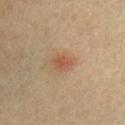<tbp_lesion>
<biopsy_status>not biopsied; imaged during a skin examination</biopsy_status>
<site>arm</site>
<lesion_size>
  <long_diameter_mm_approx>2.5</long_diameter_mm_approx>
</lesion_size>
<image>
  <source>total-body photography crop</source>
  <field_of_view_mm>15</field_of_view_mm>
</image>
<lighting>cross-polarized</lighting>
<patient>
  <sex>male</sex>
  <age_approx>40</age_approx>
</patient>
</tbp_lesion>